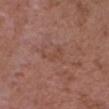The lesion was tiled from a total-body skin photograph and was not biopsied. The patient is a male aged around 50. A region of skin cropped from a whole-body photographic capture, roughly 15 mm wide. Measured at roughly 3 mm in maximum diameter. The lesion-visualizer software estimated a border-irregularity rating of about 7/10, a color-variation rating of about 1/10, and a peripheral color-asymmetry measure near 0.5. Located on the head or neck.